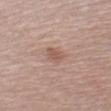biopsy_status: not biopsied; imaged during a skin examination
image:
  source: total-body photography crop
  field_of_view_mm: 15
lesion_size:
  long_diameter_mm_approx: 2.5
patient:
  sex: male
  age_approx: 55
site: left upper arm